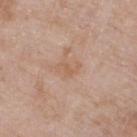Clinical impression:
Captured during whole-body skin photography for melanoma surveillance; the lesion was not biopsied.
Image and clinical context:
A close-up tile cropped from a whole-body skin photograph, about 15 mm across. Captured under white-light illumination. Located on the left lower leg. The recorded lesion diameter is about 2.5 mm. A female subject in their 70s.